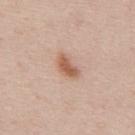automated lesion analysis: an area of roughly 5 mm², a shape eccentricity near 0.85, and a shape-asymmetry score of about 0.35 (0 = symmetric); a border-irregularity rating of about 3/10, a within-lesion color-variation index near 3/10, and radial color variation of about 1; a classifier nevus-likeness of about 95/100 and lesion-presence confidence of about 100/100 | acquisition: ~15 mm crop, total-body skin-cancer survey | body site: the back | lesion diameter: about 3.5 mm | patient: male, approximately 45 years of age | lighting: white-light illumination.